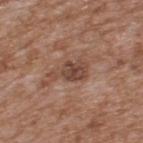| feature | finding |
|---|---|
| follow-up | catalogued during a skin exam; not biopsied |
| subject | male, about 65 years old |
| site | the upper back |
| image-analysis metrics | an eccentricity of roughly 0.85 and two-axis asymmetry of about 0.4; a mean CIELAB color near L≈45 a*≈20 b*≈26 and roughly 10 lightness units darker than nearby skin |
| lesion size | about 4 mm |
| imaging modality | total-body-photography crop, ~15 mm field of view |
| tile lighting | white-light illumination |